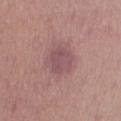This lesion was catalogued during total-body skin photography and was not selected for biopsy. The subject is a female aged 28–32. The lesion is located on the right thigh. The tile uses white-light illumination. Automated image analysis of the tile measured an area of roughly 7.5 mm², a shape eccentricity near 0.35, and two-axis asymmetry of about 0.15. And it measured a nevus-likeness score of about 0/100. The recorded lesion diameter is about 3 mm. A roughly 15 mm field-of-view crop from a total-body skin photograph.